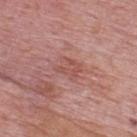Clinical impression:
This lesion was catalogued during total-body skin photography and was not selected for biopsy.
Background:
About 3 mm across. The subject is a male aged 73 to 77. The lesion-visualizer software estimated a lesion–skin lightness drop of about 7 and a normalized border contrast of about 5. The analysis additionally found an automated nevus-likeness rating near 0 out of 100 and a detector confidence of about 100 out of 100 that the crop contains a lesion. The tile uses white-light illumination. A lesion tile, about 15 mm wide, cut from a 3D total-body photograph. From the upper back.